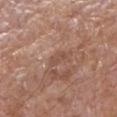No biopsy was performed on this lesion — it was imaged during a full skin examination and was not determined to be concerning. Captured under white-light illumination. Approximately 2.5 mm at its widest. This image is a 15 mm lesion crop taken from a total-body photograph. From the right forearm. A male subject approximately 80 years of age.